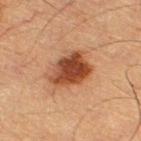site: the right thigh
lesion size: ≈5.5 mm
TBP lesion metrics: a mean CIELAB color near L≈41 a*≈23 b*≈31, about 14 CIELAB-L* units darker than the surrounding skin, and a normalized lesion–skin contrast near 10.5; a lesion-detection confidence of about 100/100
patient: male, aged approximately 85 or older
image source: total-body-photography crop, ~15 mm field of view